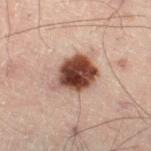Recorded during total-body skin imaging; not selected for excision or biopsy. The lesion is on the right thigh. The subject is a male aged around 50. A 15 mm close-up tile from a total-body photography series done for melanoma screening.